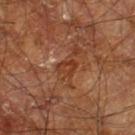workup: imaged on a skin check; not biopsied | body site: the right leg | patient: male, aged 58 to 62 | image source: ~15 mm tile from a whole-body skin photo.